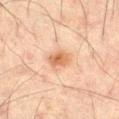automated lesion analysis = two-axis asymmetry of about 0.15; a lesion color around L≈57 a*≈21 b*≈33 in CIELAB, roughly 10 lightness units darker than nearby skin, and a normalized lesion–skin contrast near 8
body site = the right thigh
illumination = cross-polarized illumination
size = ≈3 mm
patient = male, about 45 years old
acquisition = total-body-photography crop, ~15 mm field of view